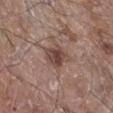  biopsy_status: not biopsied; imaged during a skin examination
  image:
    source: total-body photography crop
    field_of_view_mm: 15
  patient:
    sex: male
    age_approx: 65
  site: right lower leg
  lighting: white-light
  automated_metrics:
    border_irregularity_0_10: 3.0
    color_variation_0_10: 4.5
    peripheral_color_asymmetry: 1.5
    nevus_likeness_0_100: 10
    lesion_detection_confidence_0_100: 100
  lesion_size:
    long_diameter_mm_approx: 4.0